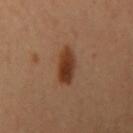workup = imaged on a skin check; not biopsied | acquisition = 15 mm crop, total-body photography | location = the left arm | patient = female, approximately 40 years of age.